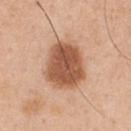– follow-up · catalogued during a skin exam; not biopsied
– location · the chest
– patient · male, in their 50s
– lighting · white-light
– image-analysis metrics · a lesion area of about 21 mm² and an eccentricity of roughly 0.6; roughly 16 lightness units darker than nearby skin and a normalized border contrast of about 10; a nevus-likeness score of about 95/100 and a detector confidence of about 100 out of 100 that the crop contains a lesion
– image source · ~15 mm crop, total-body skin-cancer survey